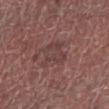Q: Was this lesion biopsied?
A: imaged on a skin check; not biopsied
Q: Who is the patient?
A: male, about 70 years old
Q: What is the lesion's diameter?
A: about 4.5 mm
Q: What is the imaging modality?
A: 15 mm crop, total-body photography
Q: Where on the body is the lesion?
A: the right forearm